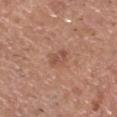Impression:
Part of a total-body skin-imaging series; this lesion was reviewed on a skin check and was not flagged for biopsy.
Image and clinical context:
Located on the head or neck. A male patient about 65 years old. Cropped from a whole-body photographic skin survey; the tile spans about 15 mm.